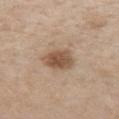lesion size: about 4 mm
site: the chest
patient: female, aged around 45
imaging modality: total-body-photography crop, ~15 mm field of view
automated metrics: a footprint of about 8 mm² and two-axis asymmetry of about 0.15